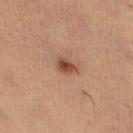notes = imaged on a skin check; not biopsied | patient = female, aged approximately 35 | anatomic site = the right lower leg | image = total-body-photography crop, ~15 mm field of view.